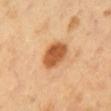biopsy status: catalogued during a skin exam; not biopsied
imaging modality: 15 mm crop, total-body photography
body site: the front of the torso
subject: male, aged 48 to 52
lesion size: ~4 mm (longest diameter)